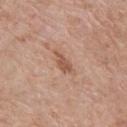Q: Is there a histopathology result?
A: imaged on a skin check; not biopsied
Q: How was this image acquired?
A: total-body-photography crop, ~15 mm field of view
Q: Where on the body is the lesion?
A: the upper back
Q: What are the patient's age and sex?
A: female, aged around 85 or older
Q: How large is the lesion?
A: about 3.5 mm